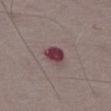workup: imaged on a skin check; not biopsied
location: the abdomen
patient: male, in their mid-60s
image: total-body-photography crop, ~15 mm field of view